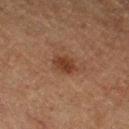notes: catalogued during a skin exam; not biopsied | subject: male, about 75 years old | anatomic site: the leg | imaging modality: 15 mm crop, total-body photography.